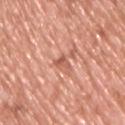Case summary:
– size · ~2.5 mm (longest diameter)
– body site · the chest
– patient · male, roughly 45 years of age
– image source · ~15 mm crop, total-body skin-cancer survey